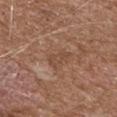Impression: Part of a total-body skin-imaging series; this lesion was reviewed on a skin check and was not flagged for biopsy. Acquisition and patient details: The lesion is on the upper back. A close-up tile cropped from a whole-body skin photograph, about 15 mm across. A female patient, about 70 years old. The recorded lesion diameter is about 3 mm.